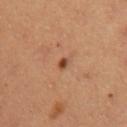Q: How was this image acquired?
A: ~15 mm crop, total-body skin-cancer survey
Q: Lesion location?
A: the left upper arm
Q: Patient demographics?
A: female, about 35 years old
Q: What lighting was used for the tile?
A: cross-polarized illumination
Q: What is the lesion's diameter?
A: ≈1.5 mm